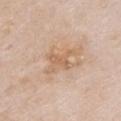Case summary:
- notes · total-body-photography surveillance lesion; no biopsy
- patient · female, about 75 years old
- image source · total-body-photography crop, ~15 mm field of view
- site · the chest
- image-analysis metrics · an average lesion color of about L≈63 a*≈18 b*≈33 (CIELAB), a lesion–skin lightness drop of about 8, and a lesion-to-skin contrast of about 5.5 (normalized; higher = more distinct); a border-irregularity rating of about 6.5/10, a color-variation rating of about 2/10, and radial color variation of about 0.5; a nevus-likeness score of about 0/100 and a detector confidence of about 100 out of 100 that the crop contains a lesion